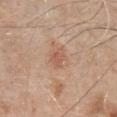{
  "biopsy_status": "not biopsied; imaged during a skin examination",
  "patient": {
    "sex": "male",
    "age_approx": 80
  },
  "image": {
    "source": "total-body photography crop",
    "field_of_view_mm": 15
  },
  "lighting": "white-light",
  "site": "chest"
}